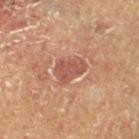– notes · no biopsy performed (imaged during a skin exam)
– site · the left lower leg
– subject · male, in their mid-60s
– image source · ~15 mm tile from a whole-body skin photo